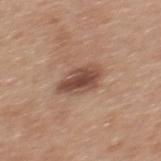No biopsy was performed on this lesion — it was imaged during a full skin examination and was not determined to be concerning. A male subject, aged around 30. Longest diameter approximately 4.5 mm. A close-up tile cropped from a whole-body skin photograph, about 15 mm across. From the mid back.